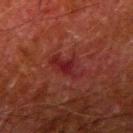Imaged during a routine full-body skin examination; the lesion was not biopsied and no histopathology is available.
A 15 mm close-up extracted from a 3D total-body photography capture.
The lesion is on the right upper arm.
The patient is a male aged 78–82.